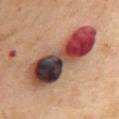Part of a total-body skin-imaging series; this lesion was reviewed on a skin check and was not flagged for biopsy. The tile uses cross-polarized illumination. The subject is a female aged 53 to 57. Measured at roughly 10.5 mm in maximum diameter. The lesion is on the mid back. A 15 mm close-up extracted from a 3D total-body photography capture.